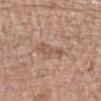Notes:
• follow-up: total-body-photography surveillance lesion; no biopsy
• anatomic site: the left forearm
• subject: male, roughly 40 years of age
• image source: ~15 mm crop, total-body skin-cancer survey